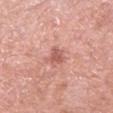Q: Is there a histopathology result?
A: imaged on a skin check; not biopsied
Q: Patient demographics?
A: female, about 40 years old
Q: What lighting was used for the tile?
A: white-light illumination
Q: What is the imaging modality?
A: ~15 mm tile from a whole-body skin photo
Q: What is the lesion's diameter?
A: ~2.5 mm (longest diameter)
Q: What is the anatomic site?
A: the left forearm